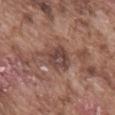Assessment: Imaged during a routine full-body skin examination; the lesion was not biopsied and no histopathology is available. Context: The patient is a male in their mid- to late 70s. A roughly 15 mm field-of-view crop from a total-body skin photograph. Located on the mid back.